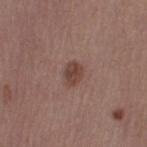workup: no biopsy performed (imaged during a skin exam)
subject: female, aged 48–52
size: ~3 mm (longest diameter)
image-analysis metrics: a border-irregularity index near 2/10, a within-lesion color-variation index near 2.5/10, and a peripheral color-asymmetry measure near 1; lesion-presence confidence of about 100/100
anatomic site: the left thigh
imaging modality: ~15 mm tile from a whole-body skin photo